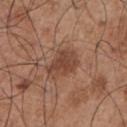Q: Was a biopsy performed?
A: total-body-photography surveillance lesion; no biopsy
Q: What are the patient's age and sex?
A: male, aged 53–57
Q: What did automated image analysis measure?
A: a lesion area of about 9.5 mm² and a shape eccentricity near 0.75; an average lesion color of about L≈44 a*≈21 b*≈29 (CIELAB) and about 9 CIELAB-L* units darker than the surrounding skin; a border-irregularity index near 2.5/10, a color-variation rating of about 2.5/10, and peripheral color asymmetry of about 0.5; an automated nevus-likeness rating near 75 out of 100
Q: Where on the body is the lesion?
A: the chest
Q: Illumination type?
A: white-light
Q: What is the lesion's diameter?
A: ≈4 mm
Q: What is the imaging modality?
A: ~15 mm tile from a whole-body skin photo